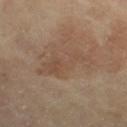The lesion was tiled from a total-body skin photograph and was not biopsied.
This is a cross-polarized tile.
Located on the right thigh.
Approximately 6.5 mm at its widest.
A male patient, aged approximately 65.
A 15 mm close-up tile from a total-body photography series done for melanoma screening.